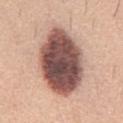Impression: Part of a total-body skin-imaging series; this lesion was reviewed on a skin check and was not flagged for biopsy. Background: A male patient, approximately 60 years of age. Automated tile analysis of the lesion measured an outline eccentricity of about 0.8 (0 = round, 1 = elongated) and two-axis asymmetry of about 0.1. The software also gave border irregularity of about 1.5 on a 0–10 scale, a color-variation rating of about 8.5/10, and radial color variation of about 2.5. And it measured a classifier nevus-likeness of about 10/100. Imaged with white-light lighting. The lesion is located on the chest. Approximately 9.5 mm at its widest. A 15 mm close-up tile from a total-body photography series done for melanoma screening.